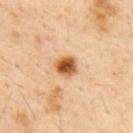Context:
Captured under cross-polarized illumination. Automated image analysis of the tile measured an area of roughly 6 mm², an eccentricity of roughly 0.35, and two-axis asymmetry of about 0.15. The software also gave a border-irregularity rating of about 1.5/10. The software also gave an automated nevus-likeness rating near 100 out of 100 and a detector confidence of about 100 out of 100 that the crop contains a lesion. The lesion's longest dimension is about 3 mm. On the upper back. Cropped from a total-body skin-imaging series; the visible field is about 15 mm. The patient is a female aged 43–47.